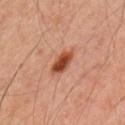notes: no biopsy performed (imaged during a skin exam) | body site: the left upper arm | subject: male, roughly 45 years of age | TBP lesion metrics: a mean CIELAB color near L≈44 a*≈27 b*≈33, roughly 15 lightness units darker than nearby skin, and a lesion-to-skin contrast of about 11 (normalized; higher = more distinct); a classifier nevus-likeness of about 100/100 and a detector confidence of about 100 out of 100 that the crop contains a lesion | image: ~15 mm crop, total-body skin-cancer survey.